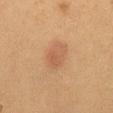Impression: Captured during whole-body skin photography for melanoma surveillance; the lesion was not biopsied. Image and clinical context: From the abdomen. This is a cross-polarized tile. The recorded lesion diameter is about 3.5 mm. A lesion tile, about 15 mm wide, cut from a 3D total-body photograph. A female patient, about 50 years old.